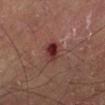The lesion was photographed on a routine skin check and not biopsied; there is no pathology result.
The lesion is on the left lower leg.
The lesion's longest dimension is about 2.5 mm.
A 15 mm close-up tile from a total-body photography series done for melanoma screening.
Imaged with cross-polarized lighting.
An algorithmic analysis of the crop reported an average lesion color of about L≈29 a*≈23 b*≈19 (CIELAB) and a lesion-to-skin contrast of about 10 (normalized; higher = more distinct). And it measured a border-irregularity rating of about 2/10 and internal color variation of about 6.5 on a 0–10 scale.
A male subject, in their mid-50s.